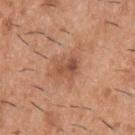Q: What kind of image is this?
A: 15 mm crop, total-body photography
Q: Patient demographics?
A: male, aged 38–42
Q: Lesion location?
A: the upper back